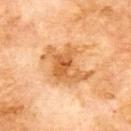Q: Was a biopsy performed?
A: no biopsy performed (imaged during a skin exam)
Q: How large is the lesion?
A: ≈6 mm
Q: How was the tile lit?
A: cross-polarized
Q: What is the imaging modality?
A: ~15 mm tile from a whole-body skin photo
Q: What is the anatomic site?
A: the upper back
Q: What are the patient's age and sex?
A: male, in their 70s